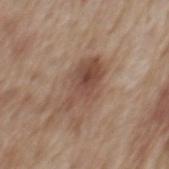Case summary:
- notes — imaged on a skin check; not biopsied
- image source — total-body-photography crop, ~15 mm field of view
- size — about 6 mm
- site — the mid back
- lighting — white-light illumination
- TBP lesion metrics — an average lesion color of about L≈48 a*≈18 b*≈27 (CIELAB), roughly 10 lightness units darker than nearby skin, and a normalized lesion–skin contrast near 7.5; a nevus-likeness score of about 55/100 and a detector confidence of about 100 out of 100 that the crop contains a lesion
- patient — male, aged 68 to 72